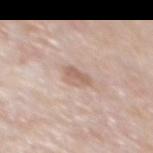workup = catalogued during a skin exam; not biopsied
site = the mid back
subject = male, aged 78 to 82
acquisition = ~15 mm crop, total-body skin-cancer survey
illumination = white-light illumination
automated lesion analysis = a mean CIELAB color near L≈62 a*≈17 b*≈26 and roughly 9 lightness units darker than nearby skin; border irregularity of about 2.5 on a 0–10 scale, internal color variation of about 2.5 on a 0–10 scale, and radial color variation of about 1